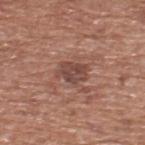biopsy status: total-body-photography surveillance lesion; no biopsy | anatomic site: the upper back | imaging modality: total-body-photography crop, ~15 mm field of view | diameter: ~3.5 mm (longest diameter) | patient: male, in their mid- to late 70s.